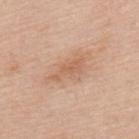notes = no biopsy performed (imaged during a skin exam) | body site = the upper back | subject = female, approximately 65 years of age | image source = total-body-photography crop, ~15 mm field of view.